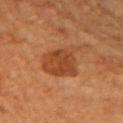Part of a total-body skin-imaging series; this lesion was reviewed on a skin check and was not flagged for biopsy. The lesion-visualizer software estimated a lesion area of about 15 mm², a shape eccentricity near 0.75, and a shape-asymmetry score of about 0.25 (0 = symmetric). The analysis additionally found a mean CIELAB color near L≈45 a*≈26 b*≈38, a lesion–skin lightness drop of about 10, and a lesion-to-skin contrast of about 8 (normalized; higher = more distinct). The patient is roughly 70 years of age. Cropped from a total-body skin-imaging series; the visible field is about 15 mm. Captured under cross-polarized illumination. On the left upper arm.